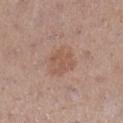{"biopsy_status": "not biopsied; imaged during a skin examination", "site": "right lower leg", "lighting": "white-light", "patient": {"sex": "female", "age_approx": 45}, "image": {"source": "total-body photography crop", "field_of_view_mm": 15}, "lesion_size": {"long_diameter_mm_approx": 4.0}}